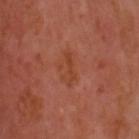No biopsy was performed on this lesion — it was imaged during a full skin examination and was not determined to be concerning. Cropped from a total-body skin-imaging series; the visible field is about 15 mm. The subject is a male in their mid- to late 50s. From the head or neck.